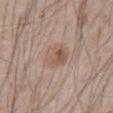• biopsy status · catalogued during a skin exam; not biopsied
• lighting · white-light
• subject · male, aged 63–67
• anatomic site · the abdomen
• lesion diameter · ≈3 mm
• TBP lesion metrics · border irregularity of about 1 on a 0–10 scale, internal color variation of about 6.5 on a 0–10 scale, and peripheral color asymmetry of about 2.5
• acquisition · 15 mm crop, total-body photography